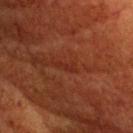| field | value |
|---|---|
| site | the head or neck |
| imaging modality | ~15 mm tile from a whole-body skin photo |
| lesion size | about 2.5 mm |
| subject | female, aged 78 to 82 |
| TBP lesion metrics | an average lesion color of about L≈22 a*≈22 b*≈24 (CIELAB), about 4 CIELAB-L* units darker than the surrounding skin, and a normalized border contrast of about 5 |
| illumination | cross-polarized |